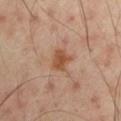Q: What is the lesion's diameter?
A: about 3 mm
Q: Lesion location?
A: the arm
Q: What is the imaging modality?
A: 15 mm crop, total-body photography
Q: Automated lesion metrics?
A: an area of roughly 5.5 mm² and two-axis asymmetry of about 0.3; a border-irregularity index near 2.5/10, a color-variation rating of about 2.5/10, and radial color variation of about 1; an automated nevus-likeness rating near 40 out of 100 and lesion-presence confidence of about 100/100
Q: What are the patient's age and sex?
A: male, aged 48–52